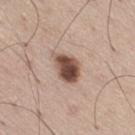workup — catalogued during a skin exam; not biopsied
lesion size — ~4.5 mm (longest diameter)
patient — male, aged around 60
automated lesion analysis — a lesion–skin lightness drop of about 18 and a normalized border contrast of about 12; a border-irregularity index near 2.5/10 and a within-lesion color-variation index near 6.5/10; a classifier nevus-likeness of about 95/100 and a lesion-detection confidence of about 100/100
image — ~15 mm tile from a whole-body skin photo
anatomic site — the right thigh
illumination — white-light